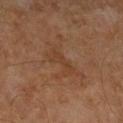biopsy status: total-body-photography surveillance lesion; no biopsy | diameter: about 3 mm | image source: ~15 mm tile from a whole-body skin photo | image-analysis metrics: a footprint of about 3.5 mm², an eccentricity of roughly 0.9, and a symmetry-axis asymmetry near 0.5; a lesion–skin lightness drop of about 5 and a lesion-to-skin contrast of about 4.5 (normalized; higher = more distinct); a border-irregularity index near 5.5/10 and radial color variation of about 0 | subject: male, about 60 years old | site: the leg | lighting: cross-polarized illumination.